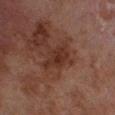Image and clinical context:
Imaged with cross-polarized lighting. The lesion is on the left lower leg. A region of skin cropped from a whole-body photographic capture, roughly 15 mm wide. The patient is a male aged 68 to 72.